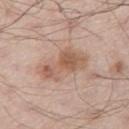Notes:
• workup · catalogued during a skin exam; not biopsied
• patient · male, aged 53–57
• acquisition · ~15 mm crop, total-body skin-cancer survey
• location · the leg